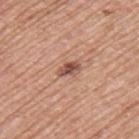Clinical impression: The lesion was photographed on a routine skin check and not biopsied; there is no pathology result. Clinical summary: An algorithmic analysis of the crop reported an area of roughly 3.5 mm², an eccentricity of roughly 0.8, and a symmetry-axis asymmetry near 0.3. The software also gave a lesion color around L≈51 a*≈23 b*≈27 in CIELAB, a lesion–skin lightness drop of about 13, and a normalized lesion–skin contrast near 9. The analysis additionally found a nevus-likeness score of about 75/100 and a detector confidence of about 100 out of 100 that the crop contains a lesion. Imaged with white-light lighting. A male patient, aged around 70. Cropped from a whole-body photographic skin survey; the tile spans about 15 mm. The lesion's longest dimension is about 2.5 mm. The lesion is located on the back.